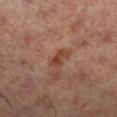Clinical impression: This lesion was catalogued during total-body skin photography and was not selected for biopsy. Context: Longest diameter approximately 2.5 mm. This image is a 15 mm lesion crop taken from a total-body photograph. This is a cross-polarized tile. From the right lower leg. A female subject roughly 60 years of age.